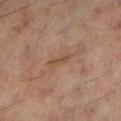Clinical impression: Imaged during a routine full-body skin examination; the lesion was not biopsied and no histopathology is available. Acquisition and patient details: Located on the left lower leg. The lesion-visualizer software estimated a classifier nevus-likeness of about 0/100 and a detector confidence of about 90 out of 100 that the crop contains a lesion. Captured under cross-polarized illumination. Measured at roughly 3 mm in maximum diameter. A male subject, aged approximately 60. A region of skin cropped from a whole-body photographic capture, roughly 15 mm wide.